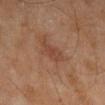biopsy status: total-body-photography surveillance lesion; no biopsy | illumination: cross-polarized illumination | patient: male, in their 60s | image: ~15 mm tile from a whole-body skin photo | diameter: ≈3 mm | site: the lower back.